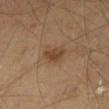Part of a total-body skin-imaging series; this lesion was reviewed on a skin check and was not flagged for biopsy.
The total-body-photography lesion software estimated a footprint of about 5.5 mm². It also reported an automated nevus-likeness rating near 70 out of 100 and a detector confidence of about 100 out of 100 that the crop contains a lesion.
From the left thigh.
A male patient roughly 70 years of age.
A region of skin cropped from a whole-body photographic capture, roughly 15 mm wide.
The lesion's longest dimension is about 3 mm.